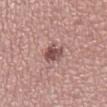Q: Is there a histopathology result?
A: no biopsy performed (imaged during a skin exam)
Q: What kind of image is this?
A: total-body-photography crop, ~15 mm field of view
Q: Patient demographics?
A: female, about 40 years old
Q: Illumination type?
A: white-light
Q: Automated lesion metrics?
A: an average lesion color of about L≈50 a*≈22 b*≈21 (CIELAB), a lesion–skin lightness drop of about 13, and a normalized border contrast of about 9
Q: Lesion location?
A: the right lower leg